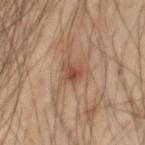This lesion was catalogued during total-body skin photography and was not selected for biopsy.
The lesion's longest dimension is about 2.5 mm.
A male patient about 60 years old.
Cropped from a total-body skin-imaging series; the visible field is about 15 mm.
Located on the chest.
This is a cross-polarized tile.